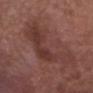Findings:
* follow-up · catalogued during a skin exam; not biopsied
* patient · male, aged 48–52
* TBP lesion metrics · internal color variation of about 4 on a 0–10 scale and peripheral color asymmetry of about 1.5
* anatomic site · the head or neck
* image source · 15 mm crop, total-body photography
* diameter · ~9 mm (longest diameter)